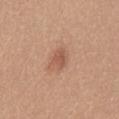The lesion was photographed on a routine skin check and not biopsied; there is no pathology result.
Captured under white-light illumination.
Cropped from a whole-body photographic skin survey; the tile spans about 15 mm.
The recorded lesion diameter is about 2.5 mm.
A female subject aged 28 to 32.
Located on the mid back.